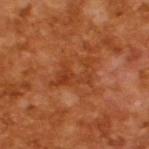follow-up: no biopsy performed (imaged during a skin exam) | image-analysis metrics: a lesion color around L≈40 a*≈29 b*≈38 in CIELAB and a lesion–skin lightness drop of about 7; a border-irregularity index near 10/10 | lesion diameter: ≈5 mm | patient: male, aged 63 to 67 | tile lighting: cross-polarized illumination | image: ~15 mm tile from a whole-body skin photo.